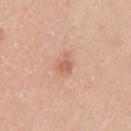This lesion was catalogued during total-body skin photography and was not selected for biopsy.